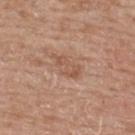Findings:
- follow-up — imaged on a skin check; not biopsied
- imaging modality — 15 mm crop, total-body photography
- lesion size — about 3.5 mm
- subject — female, in their 60s
- automated lesion analysis — an eccentricity of roughly 0.85; a border-irregularity index near 3.5/10, a color-variation rating of about 2.5/10, and radial color variation of about 0.5
- illumination — white-light illumination
- site — the back